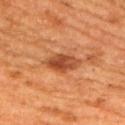follow-up — imaged on a skin check; not biopsied
patient — male, aged 63–67
diameter — ~4 mm (longest diameter)
image — 15 mm crop, total-body photography
anatomic site — the upper back
illumination — cross-polarized illumination